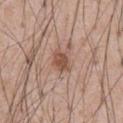biopsy_status: not biopsied; imaged during a skin examination
automated_metrics:
  nevus_likeness_0_100: 85
  lesion_detection_confidence_0_100: 100
patient:
  sex: male
  age_approx: 55
image:
  source: total-body photography crop
  field_of_view_mm: 15
site: chest
lesion_size:
  long_diameter_mm_approx: 3.0